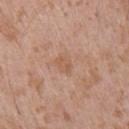The lesion was photographed on a routine skin check and not biopsied; there is no pathology result.
A lesion tile, about 15 mm wide, cut from a 3D total-body photograph.
From the right upper arm.
A male patient roughly 50 years of age.